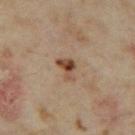Case summary:
• workup — catalogued during a skin exam; not biopsied
• patient — female, aged 33–37
• location — the leg
• tile lighting — cross-polarized
• image source — ~15 mm crop, total-body skin-cancer survey